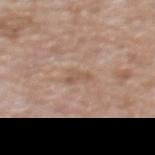notes: total-body-photography surveillance lesion; no biopsy
patient: male, aged around 60
site: the chest
image: 15 mm crop, total-body photography
lesion diameter: ~3 mm (longest diameter)